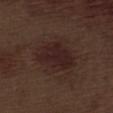The lesion was tiled from a total-body skin photograph and was not biopsied. A male subject about 70 years old. Measured at roughly 5.5 mm in maximum diameter. Located on the left thigh. A 15 mm close-up tile from a total-body photography series done for melanoma screening. Captured under white-light illumination. The lesion-visualizer software estimated a lesion area of about 16 mm², an outline eccentricity of about 0.7 (0 = round, 1 = elongated), and a symmetry-axis asymmetry near 0.25. It also reported a mean CIELAB color near L≈22 a*≈17 b*≈17 and roughly 6 lightness units darker than nearby skin. It also reported a border-irregularity rating of about 2.5/10, a within-lesion color-variation index near 2.5/10, and a peripheral color-asymmetry measure near 1. And it measured an automated nevus-likeness rating near 80 out of 100 and a detector confidence of about 100 out of 100 that the crop contains a lesion.